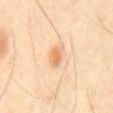Part of a total-body skin-imaging series; this lesion was reviewed on a skin check and was not flagged for biopsy.
The subject is a male aged 43–47.
Longest diameter approximately 2.5 mm.
The lesion is located on the front of the torso.
Automated image analysis of the tile measured an average lesion color of about L≈58 a*≈17 b*≈32 (CIELAB) and a lesion–skin lightness drop of about 8.
A 15 mm crop from a total-body photograph taken for skin-cancer surveillance.
This is a cross-polarized tile.